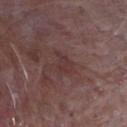Image and clinical context:
A male subject, aged 63–67. Longest diameter approximately 3 mm. The lesion-visualizer software estimated an area of roughly 4 mm², a shape eccentricity near 0.75, and a shape-asymmetry score of about 0.55 (0 = symmetric). The analysis additionally found a classifier nevus-likeness of about 0/100 and lesion-presence confidence of about 80/100. Imaged with white-light lighting. A 15 mm close-up extracted from a 3D total-body photography capture. The lesion is located on the chest.